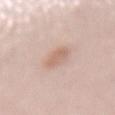{
  "biopsy_status": "not biopsied; imaged during a skin examination",
  "image": {
    "source": "total-body photography crop",
    "field_of_view_mm": 15
  },
  "lesion_size": {
    "long_diameter_mm_approx": 3.5
  },
  "patient": {
    "sex": "female",
    "age_approx": 50
  },
  "lighting": "white-light",
  "site": "mid back",
  "automated_metrics": {
    "area_mm2_approx": 5.5,
    "shape_asymmetry": 0.15,
    "border_irregularity_0_10": 2.0,
    "peripheral_color_asymmetry": 0.5,
    "nevus_likeness_0_100": 85,
    "lesion_detection_confidence_0_100": 100
  }
}